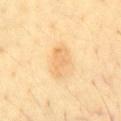{
  "biopsy_status": "not biopsied; imaged during a skin examination",
  "image": {
    "source": "total-body photography crop",
    "field_of_view_mm": 15
  },
  "site": "chest",
  "lesion_size": {
    "long_diameter_mm_approx": 4.0
  },
  "lighting": "cross-polarized",
  "patient": {
    "sex": "male",
    "age_approx": 45
  }
}